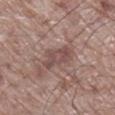Clinical impression: The lesion was tiled from a total-body skin photograph and was not biopsied. Acquisition and patient details: From the right lower leg. A roughly 15 mm field-of-view crop from a total-body skin photograph. Captured under white-light illumination. A male subject, about 70 years old.